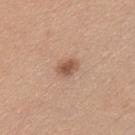Assessment: Recorded during total-body skin imaging; not selected for excision or biopsy. Clinical summary: A female subject approximately 40 years of age. The lesion-visualizer software estimated a lesion area of about 4 mm², a shape eccentricity near 0.75, and a shape-asymmetry score of about 0.25 (0 = symmetric). The software also gave an average lesion color of about L≈54 a*≈21 b*≈30 (CIELAB), a lesion–skin lightness drop of about 11, and a lesion-to-skin contrast of about 8 (normalized; higher = more distinct). It also reported an automated nevus-likeness rating near 90 out of 100 and a lesion-detection confidence of about 100/100. Cropped from a whole-body photographic skin survey; the tile spans about 15 mm. Captured under white-light illumination. Approximately 2.5 mm at its widest. From the front of the torso.